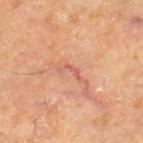Impression:
The lesion was tiled from a total-body skin photograph and was not biopsied.
Context:
On the left thigh. A roughly 15 mm field-of-view crop from a total-body skin photograph. The subject is aged 63–67.